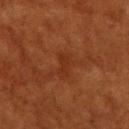Clinical impression: Imaged during a routine full-body skin examination; the lesion was not biopsied and no histopathology is available. Clinical summary: A region of skin cropped from a whole-body photographic capture, roughly 15 mm wide. From the upper back. The patient is a female roughly 80 years of age. Captured under cross-polarized illumination. An algorithmic analysis of the crop reported a lesion area of about 3 mm² and two-axis asymmetry of about 0.35. It also reported a classifier nevus-likeness of about 0/100 and lesion-presence confidence of about 100/100.